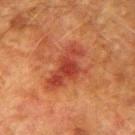Clinical impression:
Recorded during total-body skin imaging; not selected for excision or biopsy.
Context:
A male patient, aged approximately 75. The tile uses cross-polarized illumination. Approximately 6 mm at its widest. The lesion-visualizer software estimated a lesion color around L≈38 a*≈30 b*≈31 in CIELAB, roughly 9 lightness units darker than nearby skin, and a normalized lesion–skin contrast near 7.5. And it measured an automated nevus-likeness rating near 0 out of 100 and a lesion-detection confidence of about 100/100. From the right upper arm. This image is a 15 mm lesion crop taken from a total-body photograph.